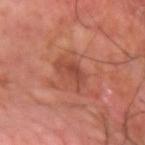Findings:
– biopsy status: catalogued during a skin exam; not biopsied
– subject: male, aged 58 to 62
– automated metrics: a footprint of about 6 mm², an outline eccentricity of about 0.8 (0 = round, 1 = elongated), and a symmetry-axis asymmetry near 0.45; an average lesion color of about L≈45 a*≈28 b*≈29 (CIELAB), about 8 CIELAB-L* units darker than the surrounding skin, and a normalized lesion–skin contrast near 6; a within-lesion color-variation index near 2.5/10 and peripheral color asymmetry of about 0.5
– tile lighting: cross-polarized illumination
– site: the arm
– lesion diameter: ≈3.5 mm
– acquisition: total-body-photography crop, ~15 mm field of view